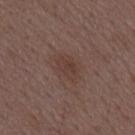Clinical impression:
This lesion was catalogued during total-body skin photography and was not selected for biopsy.
Context:
A region of skin cropped from a whole-body photographic capture, roughly 15 mm wide. Approximately 3 mm at its widest. A male patient, aged around 50. Located on the mid back.